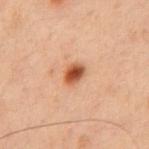| feature | finding |
|---|---|
| follow-up | no biopsy performed (imaged during a skin exam) |
| body site | the chest |
| lesion size | ≈2.5 mm |
| acquisition | 15 mm crop, total-body photography |
| automated lesion analysis | an average lesion color of about L≈41 a*≈21 b*≈30 (CIELAB), a lesion–skin lightness drop of about 13, and a normalized lesion–skin contrast near 10.5 |
| patient | male, approximately 60 years of age |